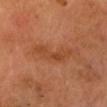<tbp_lesion>
  <biopsy_status>not biopsied; imaged during a skin examination</biopsy_status>
  <automated_metrics>
    <cielab_L>43</cielab_L>
    <cielab_a>26</cielab_a>
    <cielab_b>35</cielab_b>
    <vs_skin_darker_L>7.0</vs_skin_darker_L>
    <vs_skin_contrast_norm>6.0</vs_skin_contrast_norm>
    <border_irregularity_0_10>7.0</border_irregularity_0_10>
    <color_variation_0_10>1.5</color_variation_0_10>
    <peripheral_color_asymmetry>0.5</peripheral_color_asymmetry>
    <nevus_likeness_0_100>0</nevus_likeness_0_100>
  </automated_metrics>
  <lighting>cross-polarized</lighting>
  <site>head or neck</site>
  <patient>
    <sex>male</sex>
    <age_approx>65</age_approx>
  </patient>
  <image>
    <source>total-body photography crop</source>
    <field_of_view_mm>15</field_of_view_mm>
  </image>
  <lesion_size>
    <long_diameter_mm_approx>5.5</long_diameter_mm_approx>
  </lesion_size>
</tbp_lesion>